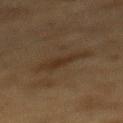{"biopsy_status": "not biopsied; imaged during a skin examination", "image": {"source": "total-body photography crop", "field_of_view_mm": 15}, "site": "mid back", "lighting": "cross-polarized", "patient": {"sex": "male", "age_approx": "85+"}, "lesion_size": {"long_diameter_mm_approx": 4.5}}